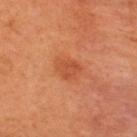The lesion was photographed on a routine skin check and not biopsied; there is no pathology result. A roughly 15 mm field-of-view crop from a total-body skin photograph. The patient is a female roughly 50 years of age. The lesion's longest dimension is about 3 mm. Automated tile analysis of the lesion measured a lesion color around L≈52 a*≈31 b*≈40 in CIELAB, a lesion–skin lightness drop of about 7, and a normalized border contrast of about 5.5. The tile uses cross-polarized illumination.